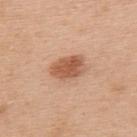<lesion>
  <biopsy_status>not biopsied; imaged during a skin examination</biopsy_status>
  <lighting>white-light</lighting>
  <image>
    <source>total-body photography crop</source>
    <field_of_view_mm>15</field_of_view_mm>
  </image>
  <site>upper back</site>
  <patient>
    <sex>male</sex>
    <age_approx>70</age_approx>
  </patient>
  <lesion_size>
    <long_diameter_mm_approx>4.0</long_diameter_mm_approx>
  </lesion_size>
</lesion>